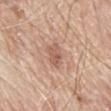{"biopsy_status": "not biopsied; imaged during a skin examination", "patient": {"sex": "male", "age_approx": 80}, "lesion_size": {"long_diameter_mm_approx": 3.0}, "automated_metrics": {"area_mm2_approx": 5.0, "eccentricity": 0.75, "shape_asymmetry": 0.25, "cielab_L": 58, "cielab_a": 21, "cielab_b": 28, "vs_skin_contrast_norm": 6.0, "border_irregularity_0_10": 2.5, "nevus_likeness_0_100": 5, "lesion_detection_confidence_0_100": 100}, "image": {"source": "total-body photography crop", "field_of_view_mm": 15}, "site": "mid back", "lighting": "white-light"}